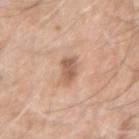biopsy status=imaged on a skin check; not biopsied
automated lesion analysis=an area of roughly 5 mm², an eccentricity of roughly 0.7, and a symmetry-axis asymmetry near 0.2; a lesion color around L≈59 a*≈21 b*≈31 in CIELAB and about 11 CIELAB-L* units darker than the surrounding skin; a nevus-likeness score of about 10/100
diameter=about 3 mm
image=~15 mm crop, total-body skin-cancer survey
body site=the right upper arm
patient=male, aged around 60